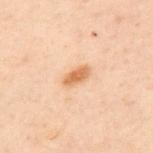Captured during whole-body skin photography for melanoma surveillance; the lesion was not biopsied.
Approximately 3 mm at its widest.
This image is a 15 mm lesion crop taken from a total-body photograph.
Automated image analysis of the tile measured a mean CIELAB color near L≈55 a*≈19 b*≈33, about 10 CIELAB-L* units darker than the surrounding skin, and a normalized border contrast of about 8. The analysis additionally found a classifier nevus-likeness of about 90/100 and a detector confidence of about 100 out of 100 that the crop contains a lesion.
The tile uses cross-polarized illumination.
A male patient, roughly 50 years of age.
Located on the back.